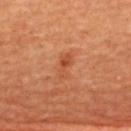Q: Was a biopsy performed?
A: total-body-photography surveillance lesion; no biopsy
Q: Where on the body is the lesion?
A: the back
Q: Patient demographics?
A: female, aged approximately 65
Q: How was this image acquired?
A: 15 mm crop, total-body photography